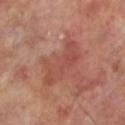workup — imaged on a skin check; not biopsied
lighting — cross-polarized illumination
image source — ~15 mm tile from a whole-body skin photo
diameter — about 5.5 mm
patient — male, aged 68–72
location — the leg
TBP lesion metrics — a footprint of about 14 mm², an eccentricity of roughly 0.85, and two-axis asymmetry of about 0.45; an average lesion color of about L≈47 a*≈26 b*≈28 (CIELAB) and a normalized border contrast of about 5; a detector confidence of about 100 out of 100 that the crop contains a lesion